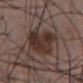workup: total-body-photography surveillance lesion; no biopsy